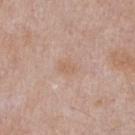| feature | finding |
|---|---|
| follow-up | catalogued during a skin exam; not biopsied |
| TBP lesion metrics | a lesion area of about 3.5 mm², an outline eccentricity of about 0.75 (0 = round, 1 = elongated), and a symmetry-axis asymmetry near 0.25; an automated nevus-likeness rating near 0 out of 100 |
| image | 15 mm crop, total-body photography |
| lighting | white-light |
| body site | the chest |
| size | ≈2.5 mm |
| subject | male, about 55 years old |